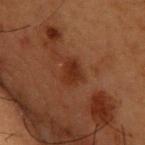Part of a total-body skin-imaging series; this lesion was reviewed on a skin check and was not flagged for biopsy.
The subject is a male in their mid- to late 50s.
This is a cross-polarized tile.
Approximately 2.5 mm at its widest.
An algorithmic analysis of the crop reported a border-irregularity rating of about 2.5/10 and a peripheral color-asymmetry measure near 0.5. The analysis additionally found a classifier nevus-likeness of about 30/100 and a detector confidence of about 100 out of 100 that the crop contains a lesion.
Located on the upper back.
A roughly 15 mm field-of-view crop from a total-body skin photograph.